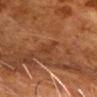Recorded during total-body skin imaging; not selected for excision or biopsy. A male subject aged around 80. Located on the chest. Longest diameter approximately 4 mm. The tile uses cross-polarized illumination. A region of skin cropped from a whole-body photographic capture, roughly 15 mm wide.